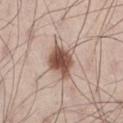Recorded during total-body skin imaging; not selected for excision or biopsy.
An algorithmic analysis of the crop reported a symmetry-axis asymmetry near 0.25. The analysis additionally found a lesion color around L≈53 a*≈18 b*≈26 in CIELAB, a lesion–skin lightness drop of about 17, and a normalized border contrast of about 11. It also reported a border-irregularity rating of about 3/10 and a within-lesion color-variation index near 4.5/10. And it measured a classifier nevus-likeness of about 95/100 and a lesion-detection confidence of about 100/100.
Approximately 4 mm at its widest.
Located on the right thigh.
A lesion tile, about 15 mm wide, cut from a 3D total-body photograph.
A male subject in their 30s.
This is a white-light tile.